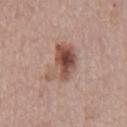This lesion was catalogued during total-body skin photography and was not selected for biopsy. The lesion is on the abdomen. The subject is a male aged around 75. Cropped from a total-body skin-imaging series; the visible field is about 15 mm.